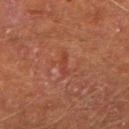workup: total-body-photography surveillance lesion; no biopsy
image-analysis metrics: an average lesion color of about L≈34 a*≈25 b*≈27 (CIELAB), roughly 5 lightness units darker than nearby skin, and a normalized border contrast of about 5; border irregularity of about 5 on a 0–10 scale, a color-variation rating of about 0/10, and radial color variation of about 0
site: the right forearm
subject: male, aged approximately 45
image: ~15 mm tile from a whole-body skin photo